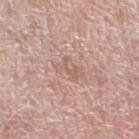This lesion was catalogued during total-body skin photography and was not selected for biopsy.
A 15 mm close-up tile from a total-body photography series done for melanoma screening.
From the left lower leg.
A female patient, aged 58–62.